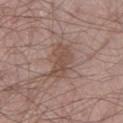Assessment:
No biopsy was performed on this lesion — it was imaged during a full skin examination and was not determined to be concerning.
Image and clinical context:
The total-body-photography lesion software estimated an area of roughly 8.5 mm², a shape eccentricity near 0.8, and two-axis asymmetry of about 0.3. On the right thigh. Measured at roughly 4 mm in maximum diameter. A 15 mm close-up tile from a total-body photography series done for melanoma screening. The subject is a male aged around 65.